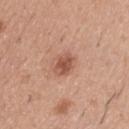An algorithmic analysis of the crop reported a lesion area of about 4.5 mm² and a shape eccentricity near 0.8. It also reported border irregularity of about 3 on a 0–10 scale, a within-lesion color-variation index near 2.5/10, and peripheral color asymmetry of about 1. It also reported an automated nevus-likeness rating near 80 out of 100 and a lesion-detection confidence of about 100/100.
A male patient aged 58–62.
Cropped from a total-body skin-imaging series; the visible field is about 15 mm.
The tile uses white-light illumination.
Measured at roughly 3 mm in maximum diameter.
From the back.